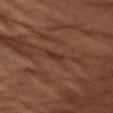Impression: The lesion was tiled from a total-body skin photograph and was not biopsied. Clinical summary: A 15 mm crop from a total-body photograph taken for skin-cancer surveillance. This is a cross-polarized tile. A female patient roughly 80 years of age. On the left upper arm. The lesion-visualizer software estimated a footprint of about 3 mm², a shape eccentricity near 0.9, and two-axis asymmetry of about 0.25. It also reported roughly 4 lightness units darker than nearby skin and a normalized lesion–skin contrast near 5.5. The analysis additionally found a border-irregularity rating of about 2.5/10, internal color variation of about 0 on a 0–10 scale, and radial color variation of about 0. And it measured a detector confidence of about 60 out of 100 that the crop contains a lesion.